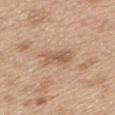  biopsy_status: not biopsied; imaged during a skin examination